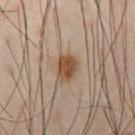No biopsy was performed on this lesion — it was imaged during a full skin examination and was not determined to be concerning. A 15 mm close-up extracted from a 3D total-body photography capture. An algorithmic analysis of the crop reported a mean CIELAB color near L≈48 a*≈19 b*≈32 and about 13 CIELAB-L* units darker than the surrounding skin. And it measured a within-lesion color-variation index near 2.5/10 and radial color variation of about 1. The analysis additionally found an automated nevus-likeness rating near 100 out of 100 and lesion-presence confidence of about 100/100. The lesion is located on the right thigh. The lesion's longest dimension is about 3 mm. Captured under cross-polarized illumination. A male subject, in their mid- to late 50s.